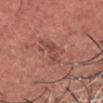notes = no biopsy performed (imaged during a skin exam) | patient = male, approximately 60 years of age | location = the head or neck | illumination = white-light illumination | acquisition = ~15 mm crop, total-body skin-cancer survey | diameter = about 6 mm.